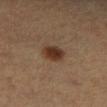Impression:
The lesion was tiled from a total-body skin photograph and was not biopsied.
Acquisition and patient details:
Located on the right lower leg. A female subject, aged around 60. A close-up tile cropped from a whole-body skin photograph, about 15 mm across.